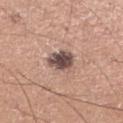Case summary:
– notes · imaged on a skin check; not biopsied
– patient · male, in their mid- to late 50s
– diameter · ~3 mm (longest diameter)
– location · the right lower leg
– imaging modality · ~15 mm crop, total-body skin-cancer survey
– lighting · white-light
– image-analysis metrics · a footprint of about 7.5 mm², an outline eccentricity of about 0.5 (0 = round, 1 = elongated), and two-axis asymmetry of about 0.2